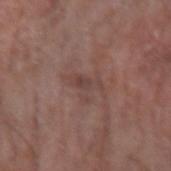A male subject in their 70s.
From the right forearm.
A lesion tile, about 15 mm wide, cut from a 3D total-body photograph.
Captured under white-light illumination.
Measured at roughly 3.5 mm in maximum diameter.